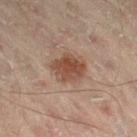<record>
<biopsy_status>not biopsied; imaged during a skin examination</biopsy_status>
<lighting>cross-polarized</lighting>
<image>
  <source>total-body photography crop</source>
  <field_of_view_mm>15</field_of_view_mm>
</image>
<site>right thigh</site>
<patient>
  <sex>male</sex>
  <age_approx>45</age_approx>
</patient>
<lesion_size>
  <long_diameter_mm_approx>4.0</long_diameter_mm_approx>
</lesion_size>
</record>